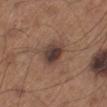Captured during whole-body skin photography for melanoma surveillance; the lesion was not biopsied.
Measured at roughly 3.5 mm in maximum diameter.
Located on the left lower leg.
Captured under white-light illumination.
The total-body-photography lesion software estimated an area of roughly 8 mm², an outline eccentricity of about 0.5 (0 = round, 1 = elongated), and a shape-asymmetry score of about 0.2 (0 = symmetric). The analysis additionally found an average lesion color of about L≈39 a*≈16 b*≈22 (CIELAB), roughly 12 lightness units darker than nearby skin, and a lesion-to-skin contrast of about 10.5 (normalized; higher = more distinct). The software also gave border irregularity of about 2 on a 0–10 scale and a within-lesion color-variation index near 6/10.
The patient is a male roughly 65 years of age.
A lesion tile, about 15 mm wide, cut from a 3D total-body photograph.